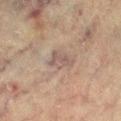Findings:
- notes · total-body-photography surveillance lesion; no biopsy
- subject · female, in their 80s
- lesion diameter · about 3.5 mm
- image source · 15 mm crop, total-body photography
- lighting · cross-polarized illumination
- site · the leg